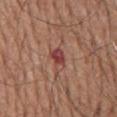  biopsy_status: not biopsied; imaged during a skin examination
  patient:
    sex: male
    age_approx: 75
  image:
    source: total-body photography crop
    field_of_view_mm: 15
  site: back
  lesion_size:
    long_diameter_mm_approx: 3.5
  lighting: white-light
  automated_metrics:
    cielab_L: 44
    cielab_a: 26
    cielab_b: 25
    vs_skin_darker_L: 10.0
    vs_skin_contrast_norm: 8.0
    color_variation_0_10: 7.0
    peripheral_color_asymmetry: 2.0